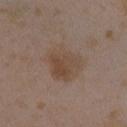Q: What kind of image is this?
A: ~15 mm tile from a whole-body skin photo
Q: Lesion location?
A: the right upper arm
Q: Who is the patient?
A: female, aged around 35
Q: Automated lesion metrics?
A: a lesion-to-skin contrast of about 6.5 (normalized; higher = more distinct)
Q: Illumination type?
A: white-light